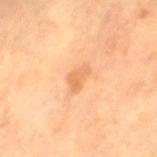Captured during whole-body skin photography for melanoma surveillance; the lesion was not biopsied.
The lesion is located on the left thigh.
Cropped from a total-body skin-imaging series; the visible field is about 15 mm.
An algorithmic analysis of the crop reported roughly 9 lightness units darker than nearby skin and a normalized border contrast of about 5.5. It also reported a nevus-likeness score of about 10/100 and a detector confidence of about 100 out of 100 that the crop contains a lesion.
A female patient in their 70s.
Captured under cross-polarized illumination.
Approximately 3 mm at its widest.